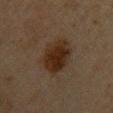notes: no biopsy performed (imaged during a skin exam) | image-analysis metrics: a lesion area of about 15 mm², a shape eccentricity near 0.75, and two-axis asymmetry of about 0.1; an average lesion color of about L≈23 a*≈14 b*≈23 (CIELAB), roughly 8 lightness units darker than nearby skin, and a normalized border contrast of about 10.5; border irregularity of about 1.5 on a 0–10 scale, internal color variation of about 4 on a 0–10 scale, and radial color variation of about 1 | tile lighting: cross-polarized illumination | image: ~15 mm tile from a whole-body skin photo | subject: female, aged around 45 | site: the back | diameter: ≈5 mm.